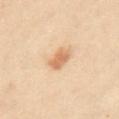Image and clinical context:
An algorithmic analysis of the crop reported a lesion area of about 5 mm² and a symmetry-axis asymmetry near 0.25. And it measured a mean CIELAB color near L≈54 a*≈17 b*≈31, about 9 CIELAB-L* units darker than the surrounding skin, and a lesion-to-skin contrast of about 7 (normalized; higher = more distinct). It also reported peripheral color asymmetry of about 1. A female patient, aged 28–32. This is a cross-polarized tile. Measured at roughly 2.5 mm in maximum diameter. The lesion is on the front of the torso. Cropped from a whole-body photographic skin survey; the tile spans about 15 mm.